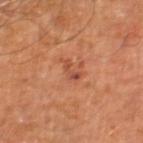Part of a total-body skin-imaging series; this lesion was reviewed on a skin check and was not flagged for biopsy. The patient is a male aged 58–62. Captured under cross-polarized illumination. Located on the right leg. A lesion tile, about 15 mm wide, cut from a 3D total-body photograph.